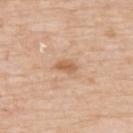Q: Is there a histopathology result?
A: no biopsy performed (imaged during a skin exam)
Q: What lighting was used for the tile?
A: white-light illumination
Q: Lesion location?
A: the upper back
Q: What kind of image is this?
A: total-body-photography crop, ~15 mm field of view
Q: What are the patient's age and sex?
A: male, in their 80s
Q: How large is the lesion?
A: ~3 mm (longest diameter)
Q: Automated lesion metrics?
A: an area of roughly 3.5 mm², a shape eccentricity near 0.85, and a shape-asymmetry score of about 0.25 (0 = symmetric); an average lesion color of about L≈61 a*≈20 b*≈35 (CIELAB) and roughly 10 lightness units darker than nearby skin